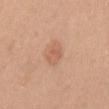{"biopsy_status": "not biopsied; imaged during a skin examination", "lesion_size": {"long_diameter_mm_approx": 3.0}, "lighting": "white-light", "image": {"source": "total-body photography crop", "field_of_view_mm": 15}, "automated_metrics": {"nevus_likeness_0_100": 45}, "patient": {"sex": "female", "age_approx": 35}, "site": "chest"}